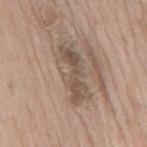The total-body-photography lesion software estimated a footprint of about 14 mm² and two-axis asymmetry of about 0.2. It also reported a classifier nevus-likeness of about 0/100 and a lesion-detection confidence of about 60/100. Cropped from a whole-body photographic skin survey; the tile spans about 15 mm. A male patient, aged around 65. The tile uses white-light illumination. The lesion is located on the mid back.